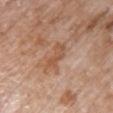Impression: No biopsy was performed on this lesion — it was imaged during a full skin examination and was not determined to be concerning. Background: On the chest. The patient is a female aged 83 or older. A close-up tile cropped from a whole-body skin photograph, about 15 mm across.